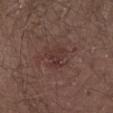The tile uses white-light illumination. Located on the front of the torso. Measured at roughly 3 mm in maximum diameter. A male subject in their mid-50s. A 15 mm crop from a total-body photograph taken for skin-cancer surveillance.